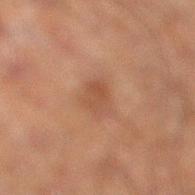Clinical impression: The lesion was photographed on a routine skin check and not biopsied; there is no pathology result. Background: The lesion's longest dimension is about 3 mm. Automated image analysis of the tile measured an outline eccentricity of about 0.7 (0 = round, 1 = elongated) and a symmetry-axis asymmetry near 0.25. It also reported a lesion color around L≈39 a*≈18 b*≈26 in CIELAB and roughly 6 lightness units darker than nearby skin. It also reported an automated nevus-likeness rating near 15 out of 100. Captured under cross-polarized illumination. The lesion is on the right lower leg. Cropped from a total-body skin-imaging series; the visible field is about 15 mm. A male subject, approximately 50 years of age.